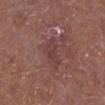follow-up = imaged on a skin check; not biopsied | image = ~15 mm crop, total-body skin-cancer survey | patient = male, roughly 75 years of age | body site = the right lower leg.